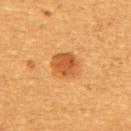follow-up: catalogued during a skin exam; not biopsied
body site: the upper back
image: total-body-photography crop, ~15 mm field of view
subject: female, aged around 55